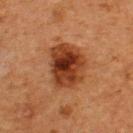Notes:
• biopsy status: no biopsy performed (imaged during a skin exam)
• image: 15 mm crop, total-body photography
• illumination: cross-polarized
• subject: male, in their mid- to late 60s
• location: the upper back
• size: about 5.5 mm
• automated lesion analysis: a lesion color around L≈33 a*≈24 b*≈32 in CIELAB and about 14 CIELAB-L* units darker than the surrounding skin; a border-irregularity index near 2/10, internal color variation of about 7.5 on a 0–10 scale, and a peripheral color-asymmetry measure near 2.5